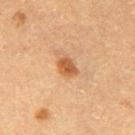This lesion was catalogued during total-body skin photography and was not selected for biopsy. A female patient aged around 60. The lesion is on the leg. Measured at roughly 2.5 mm in maximum diameter. This image is a 15 mm lesion crop taken from a total-body photograph.